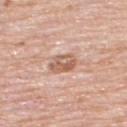Part of a total-body skin-imaging series; this lesion was reviewed on a skin check and was not flagged for biopsy.
Located on the upper back.
The subject is a male aged 78–82.
This is a white-light tile.
A 15 mm crop from a total-body photograph taken for skin-cancer surveillance.